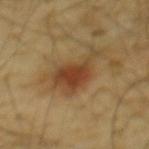* workup · no biopsy performed (imaged during a skin exam)
* subject · male, aged around 65
* diameter · ~5.5 mm (longest diameter)
* site · the mid back
* acquisition · total-body-photography crop, ~15 mm field of view
* lighting · cross-polarized